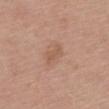acquisition: ~15 mm tile from a whole-body skin photo
location: the chest
TBP lesion metrics: an area of roughly 4 mm², an outline eccentricity of about 0.85 (0 = round, 1 = elongated), and a shape-asymmetry score of about 0.3 (0 = symmetric); an average lesion color of about L≈56 a*≈20 b*≈30 (CIELAB) and a normalized lesion–skin contrast near 5; border irregularity of about 3.5 on a 0–10 scale, a color-variation rating of about 1.5/10, and peripheral color asymmetry of about 0.5
diameter: ~3 mm (longest diameter)
patient: female, aged 63 to 67